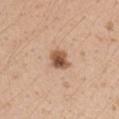Q: Is there a histopathology result?
A: no biopsy performed (imaged during a skin exam)
Q: What kind of image is this?
A: 15 mm crop, total-body photography
Q: Lesion location?
A: the left upper arm
Q: Patient demographics?
A: female, in their 30s